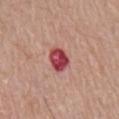Recorded during total-body skin imaging; not selected for excision or biopsy.
On the chest.
Cropped from a total-body skin-imaging series; the visible field is about 15 mm.
A male patient aged 78–82.